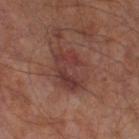Q: Was a biopsy performed?
A: imaged on a skin check; not biopsied
Q: How was this image acquired?
A: ~15 mm crop, total-body skin-cancer survey
Q: How large is the lesion?
A: ≈5 mm
Q: How was the tile lit?
A: cross-polarized
Q: What are the patient's age and sex?
A: male, in their mid-60s
Q: Where on the body is the lesion?
A: the right thigh